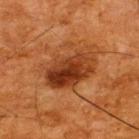Imaged during a routine full-body skin examination; the lesion was not biopsied and no histopathology is available.
Cropped from a total-body skin-imaging series; the visible field is about 15 mm.
From the upper back.
A male subject, aged 63–67.